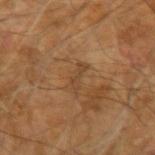<tbp_lesion>
  <biopsy_status>not biopsied; imaged during a skin examination</biopsy_status>
  <lighting>cross-polarized</lighting>
  <image>
    <source>total-body photography crop</source>
    <field_of_view_mm>15</field_of_view_mm>
  </image>
  <automated_metrics>
    <cielab_L>42</cielab_L>
    <cielab_a>17</cielab_a>
    <cielab_b>33</cielab_b>
    <vs_skin_darker_L>6.0</vs_skin_darker_L>
    <vs_skin_contrast_norm>5.5</vs_skin_contrast_norm>
  </automated_metrics>
  <patient>
    <sex>male</sex>
    <age_approx>60</age_approx>
  </patient>
  <site>left arm</site>
  <lesion_size>
    <long_diameter_mm_approx>3.5</long_diameter_mm_approx>
  </lesion_size>
</tbp_lesion>